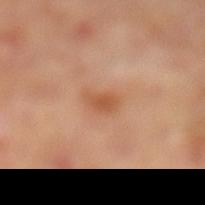Recorded during total-body skin imaging; not selected for excision or biopsy. A male subject about 50 years old. Measured at roughly 2.5 mm in maximum diameter. A 15 mm crop from a total-body photograph taken for skin-cancer surveillance. The tile uses cross-polarized illumination. The lesion is located on the left lower leg.